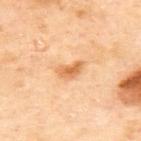<lesion>
  <biopsy_status>not biopsied; imaged during a skin examination</biopsy_status>
  <image>
    <source>total-body photography crop</source>
    <field_of_view_mm>15</field_of_view_mm>
  </image>
  <lighting>cross-polarized</lighting>
  <patient>
    <sex>female</sex>
    <age_approx>60</age_approx>
  </patient>
  <lesion_size>
    <long_diameter_mm_approx>3.0</long_diameter_mm_approx>
  </lesion_size>
  <automated_metrics>
    <cielab_L>67</cielab_L>
    <cielab_a>25</cielab_a>
    <cielab_b>44</cielab_b>
    <border_irregularity_0_10>3.5</border_irregularity_0_10>
    <color_variation_0_10>2.5</color_variation_0_10>
    <peripheral_color_asymmetry>0.5</peripheral_color_asymmetry>
  </automated_metrics>
  <site>upper back</site>
</lesion>